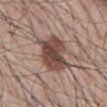Notes:
* notes · imaged on a skin check; not biopsied
* automated lesion analysis · an eccentricity of roughly 0.8 and two-axis asymmetry of about 0.25; a lesion color around L≈46 a*≈18 b*≈23 in CIELAB, about 14 CIELAB-L* units darker than the surrounding skin, and a normalized lesion–skin contrast near 10
* site · the mid back
* subject · male, roughly 45 years of age
* lesion diameter · ≈6 mm
* image · ~15 mm tile from a whole-body skin photo